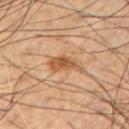{
  "biopsy_status": "not biopsied; imaged during a skin examination",
  "site": "chest",
  "lesion_size": {
    "long_diameter_mm_approx": 4.0
  },
  "image": {
    "source": "total-body photography crop",
    "field_of_view_mm": 15
  },
  "patient": {
    "sex": "male",
    "age_approx": 45
  }
}